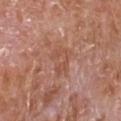Imaged during a routine full-body skin examination; the lesion was not biopsied and no histopathology is available. The recorded lesion diameter is about 4 mm. A male subject in their mid-60s. This is a white-light tile. The lesion is on the front of the torso. A 15 mm crop from a total-body photograph taken for skin-cancer surveillance.